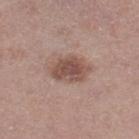Recorded during total-body skin imaging; not selected for excision or biopsy. The total-body-photography lesion software estimated a border-irregularity rating of about 2/10, internal color variation of about 3.5 on a 0–10 scale, and peripheral color asymmetry of about 1. And it measured a nevus-likeness score of about 50/100 and a lesion-detection confidence of about 100/100. The patient is a female aged 48–52. Cropped from a total-body skin-imaging series; the visible field is about 15 mm. The lesion's longest dimension is about 4.5 mm. Imaged with white-light lighting. Located on the right thigh.